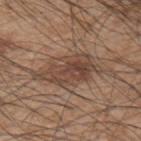follow-up: imaged on a skin check; not biopsied | patient: male, roughly 45 years of age | body site: the left upper arm | lesion size: ~7 mm (longest diameter) | imaging modality: ~15 mm crop, total-body skin-cancer survey.